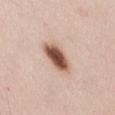The lesion was photographed on a routine skin check and not biopsied; there is no pathology result. The lesion is on the chest. A roughly 15 mm field-of-view crop from a total-body skin photograph. Imaged with white-light lighting. A male patient, aged 48 to 52.